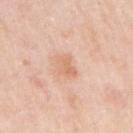lesion size = about 3 mm | site = the right upper arm | subject = female, about 65 years old | image = ~15 mm tile from a whole-body skin photo | TBP lesion metrics = an area of roughly 5 mm², an eccentricity of roughly 0.65, and a symmetry-axis asymmetry near 0.3; a within-lesion color-variation index near 2.5/10 and a peripheral color-asymmetry measure near 1 | tile lighting = white-light.